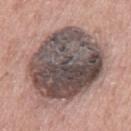The lesion was tiled from a total-body skin photograph and was not biopsied. The lesion's longest dimension is about 9.5 mm. A male patient, approximately 60 years of age. Located on the mid back. Imaged with white-light lighting. A 15 mm close-up extracted from a 3D total-body photography capture.